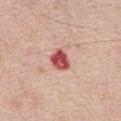Captured during whole-body skin photography for melanoma surveillance; the lesion was not biopsied.